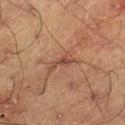Recorded during total-body skin imaging; not selected for excision or biopsy. The lesion is located on the right lower leg. The patient is a male aged 58 to 62. A roughly 15 mm field-of-view crop from a total-body skin photograph.